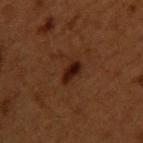Notes:
- notes: imaged on a skin check; not biopsied